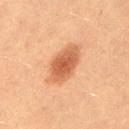The lesion was photographed on a routine skin check and not biopsied; there is no pathology result. A close-up tile cropped from a whole-body skin photograph, about 15 mm across. Located on the left thigh. About 5.5 mm across. Imaged with cross-polarized lighting. A female patient, aged 18 to 22.